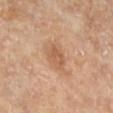patient = male, in their mid-80s or older
image source = ~15 mm tile from a whole-body skin photo
tile lighting = cross-polarized
site = the left lower leg
diameter = about 3 mm